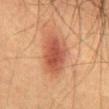Q: Who is the patient?
A: male, about 50 years old
Q: Where on the body is the lesion?
A: the abdomen
Q: How was this image acquired?
A: total-body-photography crop, ~15 mm field of view